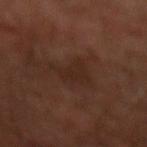The lesion was tiled from a total-body skin photograph and was not biopsied.
A male patient, in their 60s.
The lesion's longest dimension is about 3.5 mm.
The total-body-photography lesion software estimated a border-irregularity rating of about 4.5/10, a within-lesion color-variation index near 1.5/10, and radial color variation of about 0.5. The software also gave an automated nevus-likeness rating near 0 out of 100.
A 15 mm close-up tile from a total-body photography series done for melanoma screening.
This is a cross-polarized tile.
Located on the left forearm.